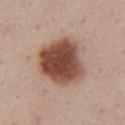biopsy_status: not biopsied; imaged during a skin examination
lighting: white-light
automated_metrics:
  border_irregularity_0_10: 1.5
  color_variation_0_10: 6.5
  peripheral_color_asymmetry: 2.0
  nevus_likeness_0_100: 100
lesion_size:
  long_diameter_mm_approx: 6.0
image:
  source: total-body photography crop
  field_of_view_mm: 15
patient:
  sex: female
  age_approx: 55
site: chest